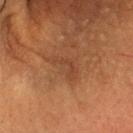Image and clinical context:
The tile uses cross-polarized illumination. Approximately 4 mm at its widest. A male patient aged 53 to 57. A close-up tile cropped from a whole-body skin photograph, about 15 mm across. The lesion is located on the head or neck. The total-body-photography lesion software estimated a shape-asymmetry score of about 0.6 (0 = symmetric). The software also gave an average lesion color of about L≈37 a*≈20 b*≈29 (CIELAB) and a normalized lesion–skin contrast near 5. It also reported a border-irregularity rating of about 6.5/10, internal color variation of about 2 on a 0–10 scale, and a peripheral color-asymmetry measure near 0.5. And it measured a classifier nevus-likeness of about 0/100 and lesion-presence confidence of about 100/100.